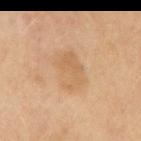This lesion was catalogued during total-body skin photography and was not selected for biopsy. The subject is a male approximately 70 years of age. On the mid back. The lesion's longest dimension is about 5 mm. A 15 mm close-up tile from a total-body photography series done for melanoma screening.